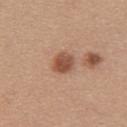Q: Was a biopsy performed?
A: no biopsy performed (imaged during a skin exam)
Q: What is the anatomic site?
A: the upper back
Q: What did automated image analysis measure?
A: about 13 CIELAB-L* units darker than the surrounding skin; a border-irregularity index near 1.5/10, a color-variation rating of about 3.5/10, and radial color variation of about 1.5; a detector confidence of about 100 out of 100 that the crop contains a lesion
Q: Who is the patient?
A: female, aged approximately 35
Q: How large is the lesion?
A: ≈2.5 mm
Q: What is the imaging modality?
A: ~15 mm tile from a whole-body skin photo
Q: What lighting was used for the tile?
A: white-light